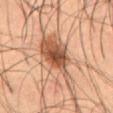Cropped from a whole-body photographic skin survey; the tile spans about 15 mm.
The patient is a male in their mid-50s.
The lesion is located on the abdomen.
The recorded lesion diameter is about 9 mm.
This is a cross-polarized tile.